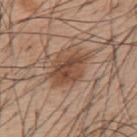Clinical impression:
No biopsy was performed on this lesion — it was imaged during a full skin examination and was not determined to be concerning.
Acquisition and patient details:
Approximately 5 mm at its widest. On the upper back. A close-up tile cropped from a whole-body skin photograph, about 15 mm across. A male subject, in their mid-50s.